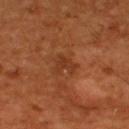biopsy status — catalogued during a skin exam; not biopsied | lesion size — ~3.5 mm (longest diameter) | automated metrics — a symmetry-axis asymmetry near 0.5; an average lesion color of about L≈34 a*≈24 b*≈33 (CIELAB), a lesion–skin lightness drop of about 6, and a normalized lesion–skin contrast near 5.5; a peripheral color-asymmetry measure near 0.5 | image — ~15 mm crop, total-body skin-cancer survey | subject — male, aged 53 to 57 | anatomic site — the upper back | illumination — cross-polarized.